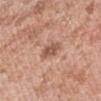Imaged during a routine full-body skin examination; the lesion was not biopsied and no histopathology is available. This is a white-light tile. The lesion is on the left upper arm. A male subject about 40 years old. Cropped from a whole-body photographic skin survey; the tile spans about 15 mm.